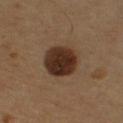Notes:
* notes — catalogued during a skin exam; not biopsied
* image — ~15 mm tile from a whole-body skin photo
* body site — the right upper arm
* illumination — cross-polarized
* subject — male, aged approximately 55